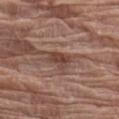The total-body-photography lesion software estimated an automated nevus-likeness rating near 10 out of 100 and a detector confidence of about 95 out of 100 that the crop contains a lesion. Measured at roughly 3 mm in maximum diameter. Cropped from a whole-body photographic skin survey; the tile spans about 15 mm. The tile uses white-light illumination. From the left forearm. The subject is a male aged 78 to 82.